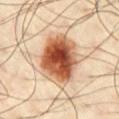The lesion was photographed on a routine skin check and not biopsied; there is no pathology result. A male patient, about 65 years old. Approximately 6.5 mm at its widest. A region of skin cropped from a whole-body photographic capture, roughly 15 mm wide. On the front of the torso.